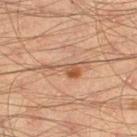biopsy status: catalogued during a skin exam; not biopsied | location: the leg | acquisition: total-body-photography crop, ~15 mm field of view | subject: male, aged around 45.